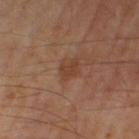Imaged during a routine full-body skin examination; the lesion was not biopsied and no histopathology is available. A male patient aged 63 to 67. From the left leg. Imaged with cross-polarized lighting. A roughly 15 mm field-of-view crop from a total-body skin photograph. About 2.5 mm across.